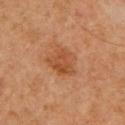Assessment: Captured during whole-body skin photography for melanoma surveillance; the lesion was not biopsied. Image and clinical context: The lesion is on the right upper arm. The lesion's longest dimension is about 3.5 mm. The tile uses cross-polarized illumination. A male patient, approximately 60 years of age. This image is a 15 mm lesion crop taken from a total-body photograph.